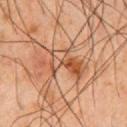Imaged during a routine full-body skin examination; the lesion was not biopsied and no histopathology is available.
A close-up tile cropped from a whole-body skin photograph, about 15 mm across.
Longest diameter approximately 5.5 mm.
A male patient, aged around 65.
The lesion is on the chest.